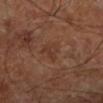Imaged during a routine full-body skin examination; the lesion was not biopsied and no histopathology is available.
An algorithmic analysis of the crop reported a shape eccentricity near 0.7 and a symmetry-axis asymmetry near 0.45. The software also gave a border-irregularity rating of about 4.5/10, a color-variation rating of about 2.5/10, and radial color variation of about 1. The software also gave a nevus-likeness score of about 0/100 and a lesion-detection confidence of about 100/100.
Cropped from a total-body skin-imaging series; the visible field is about 15 mm.
The tile uses cross-polarized illumination.
Longest diameter approximately 3 mm.
Located on the leg.
The patient is aged around 65.